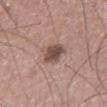Notes:
- workup: imaged on a skin check; not biopsied
- illumination: white-light illumination
- lesion diameter: about 3.5 mm
- image: 15 mm crop, total-body photography
- anatomic site: the left thigh
- subject: male, about 75 years old